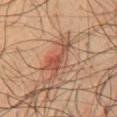Measured at roughly 5.5 mm in maximum diameter.
Located on the chest.
A male patient, in their mid-60s.
A region of skin cropped from a whole-body photographic capture, roughly 15 mm wide.